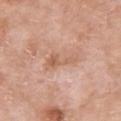{"biopsy_status": "not biopsied; imaged during a skin examination", "patient": {"sex": "female", "age_approx": 75}, "lighting": "white-light", "lesion_size": {"long_diameter_mm_approx": 4.5}, "site": "chest", "automated_metrics": {"area_mm2_approx": 5.5, "eccentricity": 0.9, "shape_asymmetry": 0.6, "color_variation_0_10": 2.5, "peripheral_color_asymmetry": 0.5, "nevus_likeness_0_100": 0, "lesion_detection_confidence_0_100": 100}, "image": {"source": "total-body photography crop", "field_of_view_mm": 15}}